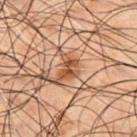The lesion was photographed on a routine skin check and not biopsied; there is no pathology result. The lesion is on the chest. Cropped from a total-body skin-imaging series; the visible field is about 15 mm. The subject is a male aged approximately 50.